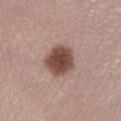follow-up: no biopsy performed (imaged during a skin exam); patient: female, in their 20s; body site: the leg; acquisition: total-body-photography crop, ~15 mm field of view.